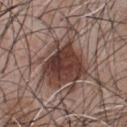Q: What is the anatomic site?
A: the chest
Q: What is the imaging modality?
A: ~15 mm tile from a whole-body skin photo
Q: Who is the patient?
A: male, aged 43–47
Q: Automated lesion metrics?
A: a lesion color around L≈41 a*≈18 b*≈22 in CIELAB, roughly 13 lightness units darker than nearby skin, and a lesion-to-skin contrast of about 10.5 (normalized; higher = more distinct); a color-variation rating of about 6.5/10 and peripheral color asymmetry of about 2